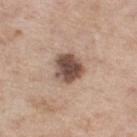{"automated_metrics": {"area_mm2_approx": 10.0, "eccentricity": 0.3, "shape_asymmetry": 0.2, "cielab_L": 50, "cielab_a": 17, "cielab_b": 24, "vs_skin_contrast_norm": 11.5, "nevus_likeness_0_100": 65, "lesion_detection_confidence_0_100": 100}, "site": "right thigh", "image": {"source": "total-body photography crop", "field_of_view_mm": 15}, "lighting": "white-light", "patient": {"sex": "female", "age_approx": 55}}